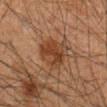biopsy_status: not biopsied; imaged during a skin examination
lighting: cross-polarized
automated_metrics:
  vs_skin_darker_L: 8.0
  vs_skin_contrast_norm: 7.5
  border_irregularity_0_10: 2.5
  color_variation_0_10: 4.5
  lesion_detection_confidence_0_100: 100
patient:
  sex: male
  age_approx: 60
lesion_size:
  long_diameter_mm_approx: 4.5
image:
  source: total-body photography crop
  field_of_view_mm: 15
site: mid back